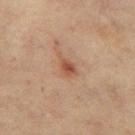biopsy status: catalogued during a skin exam; not biopsied | automated metrics: a lesion color around L≈46 a*≈19 b*≈28 in CIELAB, about 8 CIELAB-L* units darker than the surrounding skin, and a normalized lesion–skin contrast near 7 | image: ~15 mm tile from a whole-body skin photo | site: the left thigh | diameter: ~3.5 mm (longest diameter) | lighting: cross-polarized | patient: female, roughly 55 years of age.